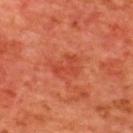{
  "biopsy_status": "not biopsied; imaged during a skin examination",
  "lesion_size": {
    "long_diameter_mm_approx": 3.0
  },
  "patient": {
    "sex": "male",
    "age_approx": 65
  },
  "site": "upper back",
  "image": {
    "source": "total-body photography crop",
    "field_of_view_mm": 15
  },
  "automated_metrics": {
    "area_mm2_approx": 4.0,
    "eccentricity": 0.8,
    "shape_asymmetry": 0.65,
    "vs_skin_darker_L": 6.0,
    "border_irregularity_0_10": 9.0,
    "peripheral_color_asymmetry": 0.0
  },
  "lighting": "cross-polarized"
}